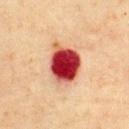Impression:
Captured during whole-body skin photography for melanoma surveillance; the lesion was not biopsied.
Acquisition and patient details:
The recorded lesion diameter is about 5 mm. A male patient, aged around 60. Imaged with cross-polarized lighting. Cropped from a total-body skin-imaging series; the visible field is about 15 mm. From the chest. Automated image analysis of the tile measured an area of roughly 15 mm², an outline eccentricity of about 0.65 (0 = round, 1 = elongated), and a shape-asymmetry score of about 0.25 (0 = symmetric). The analysis additionally found a border-irregularity rating of about 2/10, internal color variation of about 7.5 on a 0–10 scale, and radial color variation of about 1.5. The software also gave a nevus-likeness score of about 0/100 and a lesion-detection confidence of about 100/100.